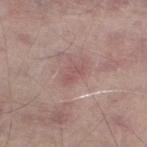follow-up: total-body-photography surveillance lesion; no biopsy | patient: male, about 65 years old | location: the left lower leg | illumination: white-light | acquisition: ~15 mm tile from a whole-body skin photo | lesion diameter: ≈2.5 mm | automated metrics: an outline eccentricity of about 0.85 (0 = round, 1 = elongated) and two-axis asymmetry of about 0.35; a mean CIELAB color near L≈53 a*≈21 b*≈20, roughly 7 lightness units darker than nearby skin, and a normalized lesion–skin contrast near 5; radial color variation of about 0; a detector confidence of about 100 out of 100 that the crop contains a lesion.